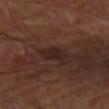Impression: Part of a total-body skin-imaging series; this lesion was reviewed on a skin check and was not flagged for biopsy. Clinical summary: Captured under cross-polarized illumination. Located on the right forearm. This image is a 15 mm lesion crop taken from a total-body photograph. The lesion's longest dimension is about 3 mm.